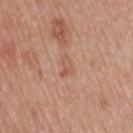Impression: The lesion was photographed on a routine skin check and not biopsied; there is no pathology result. Clinical summary: Imaged with white-light lighting. The lesion is on the upper back. A male subject, about 60 years old. About 2.5 mm across. Cropped from a whole-body photographic skin survey; the tile spans about 15 mm.